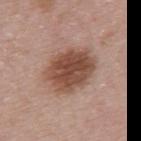Notes:
– workup · total-body-photography surveillance lesion; no biopsy
– anatomic site · the upper back
– subject · female, in their 30s
– imaging modality · ~15 mm crop, total-body skin-cancer survey